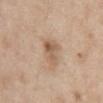Impression: The lesion was tiled from a total-body skin photograph and was not biopsied. Context: Located on the abdomen. A region of skin cropped from a whole-body photographic capture, roughly 15 mm wide. The subject is a female aged approximately 40. Imaged with white-light lighting.